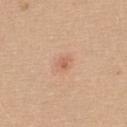Q: Was this lesion biopsied?
A: imaged on a skin check; not biopsied
Q: How was this image acquired?
A: ~15 mm crop, total-body skin-cancer survey
Q: Automated lesion metrics?
A: a lesion color around L≈61 a*≈23 b*≈32 in CIELAB, roughly 9 lightness units darker than nearby skin, and a normalized border contrast of about 5.5; a nevus-likeness score of about 10/100 and a detector confidence of about 100 out of 100 that the crop contains a lesion
Q: Patient demographics?
A: female, about 20 years old
Q: What is the anatomic site?
A: the upper back
Q: How was the tile lit?
A: white-light
Q: How large is the lesion?
A: ~1.5 mm (longest diameter)